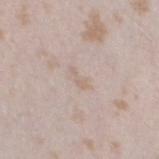workup: catalogued during a skin exam; not biopsied
patient: female, aged 23–27
location: the left thigh
tile lighting: white-light illumination
lesion size: about 2.5 mm
image source: ~15 mm tile from a whole-body skin photo